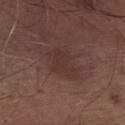Q: Is there a histopathology result?
A: total-body-photography surveillance lesion; no biopsy
Q: How large is the lesion?
A: ≈4.5 mm
Q: What is the anatomic site?
A: the front of the torso
Q: What are the patient's age and sex?
A: male, about 80 years old
Q: How was the tile lit?
A: white-light
Q: What kind of image is this?
A: ~15 mm crop, total-body skin-cancer survey
Q: What did automated image analysis measure?
A: border irregularity of about 4 on a 0–10 scale, a color-variation rating of about 2/10, and radial color variation of about 0.5